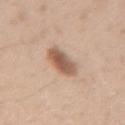Impression: Part of a total-body skin-imaging series; this lesion was reviewed on a skin check and was not flagged for biopsy. Background: The lesion is on the arm. A female subject, aged 23 to 27. A 15 mm crop from a total-body photograph taken for skin-cancer surveillance. The lesion's longest dimension is about 3.5 mm. Imaged with white-light lighting. An algorithmic analysis of the crop reported about 14 CIELAB-L* units darker than the surrounding skin. The analysis additionally found a classifier nevus-likeness of about 90/100 and a detector confidence of about 100 out of 100 that the crop contains a lesion.